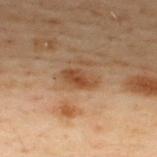Clinical impression: No biopsy was performed on this lesion — it was imaged during a full skin examination and was not determined to be concerning. Context: A male patient, aged around 50. The lesion is located on the upper back. This is a cross-polarized tile. A lesion tile, about 15 mm wide, cut from a 3D total-body photograph.